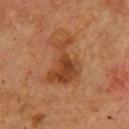acquisition = 15 mm crop, total-body photography
site = the chest
subject = male, approximately 75 years of age